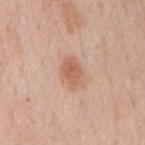The lesion was photographed on a routine skin check and not biopsied; there is no pathology result. A male patient, approximately 60 years of age. Located on the back. A 15 mm close-up extracted from a 3D total-body photography capture. An algorithmic analysis of the crop reported a footprint of about 6.5 mm² and a shape-asymmetry score of about 0.25 (0 = symmetric). The software also gave border irregularity of about 2.5 on a 0–10 scale, internal color variation of about 3 on a 0–10 scale, and peripheral color asymmetry of about 1. It also reported a detector confidence of about 100 out of 100 that the crop contains a lesion. The tile uses white-light illumination.